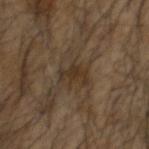notes = imaged on a skin check; not biopsied | automated lesion analysis = a footprint of about 5 mm², an eccentricity of roughly 0.85, and a symmetry-axis asymmetry near 0.4; a border-irregularity index near 5.5/10 and internal color variation of about 1.5 on a 0–10 scale; a nevus-likeness score of about 5/100 | patient = male, approximately 55 years of age | lesion diameter = about 3.5 mm | image source = 15 mm crop, total-body photography | anatomic site = the head or neck | tile lighting = cross-polarized.